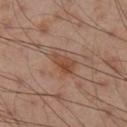follow-up — catalogued during a skin exam; not biopsied | subject — male, aged approximately 55 | image source — total-body-photography crop, ~15 mm field of view | location — the right lower leg.